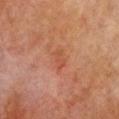Imaged during a routine full-body skin examination; the lesion was not biopsied and no histopathology is available. A 15 mm close-up tile from a total-body photography series done for melanoma screening. Automated tile analysis of the lesion measured an area of roughly 3 mm², an outline eccentricity of about 0.85 (0 = round, 1 = elongated), and two-axis asymmetry of about 0.35. The analysis additionally found a border-irregularity index near 3.5/10 and radial color variation of about 0.5. The analysis additionally found an automated nevus-likeness rating near 0 out of 100 and a detector confidence of about 100 out of 100 that the crop contains a lesion. On the chest. Measured at roughly 2.5 mm in maximum diameter. Captured under cross-polarized illumination. A female subject, roughly 80 years of age.